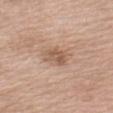This lesion was catalogued during total-body skin photography and was not selected for biopsy.
Located on the left upper arm.
A 15 mm crop from a total-body photograph taken for skin-cancer surveillance.
This is a white-light tile.
A female patient, aged 53–57.
About 3 mm across.